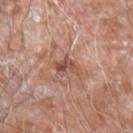Impression: No biopsy was performed on this lesion — it was imaged during a full skin examination and was not determined to be concerning. Clinical summary: Cropped from a total-body skin-imaging series; the visible field is about 15 mm. A male patient about 60 years old. Longest diameter approximately 3 mm. The lesion is located on the left forearm. Imaged with white-light lighting.